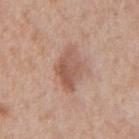Clinical summary:
The tile uses white-light illumination. Automated image analysis of the tile measured a lesion color around L≈55 a*≈21 b*≈28 in CIELAB, a lesion–skin lightness drop of about 10, and a normalized lesion–skin contrast near 7. The analysis additionally found a nevus-likeness score of about 10/100 and a lesion-detection confidence of about 100/100. The recorded lesion diameter is about 4.5 mm. Located on the mid back. A 15 mm close-up tile from a total-body photography series done for melanoma screening. The patient is a male aged 63 to 67.